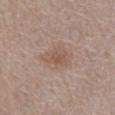Clinical impression: The lesion was photographed on a routine skin check and not biopsied; there is no pathology result. Clinical summary: From the abdomen. The patient is a female in their 80s. A 15 mm crop from a total-body photograph taken for skin-cancer surveillance. This is a white-light tile.